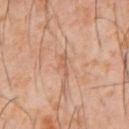Imaged during a routine full-body skin examination; the lesion was not biopsied and no histopathology is available. The patient is a male aged 58–62. This image is a 15 mm lesion crop taken from a total-body photograph. On the abdomen. Imaged with cross-polarized lighting. The recorded lesion diameter is about 2.5 mm.